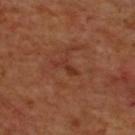notes: total-body-photography surveillance lesion; no biopsy | imaging modality: total-body-photography crop, ~15 mm field of view | automated lesion analysis: a lesion color around L≈32 a*≈25 b*≈29 in CIELAB, roughly 6 lightness units darker than nearby skin, and a normalized lesion–skin contrast near 6; internal color variation of about 0 on a 0–10 scale and radial color variation of about 0; a classifier nevus-likeness of about 0/100 and a lesion-detection confidence of about 100/100 | site: the back | lesion diameter: ~3 mm (longest diameter) | patient: male, aged 68–72.